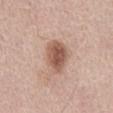Case summary:
– automated metrics · an average lesion color of about L≈55 a*≈20 b*≈27 (CIELAB), a lesion–skin lightness drop of about 14, and a normalized lesion–skin contrast near 9; a border-irregularity rating of about 2/10, internal color variation of about 4 on a 0–10 scale, and peripheral color asymmetry of about 1; an automated nevus-likeness rating near 95 out of 100 and a lesion-detection confidence of about 100/100
– illumination · white-light illumination
– lesion diameter · ≈4.5 mm
– image source · total-body-photography crop, ~15 mm field of view
– site · the front of the torso
– patient · male, in their mid- to late 60s